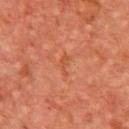image — 15 mm crop, total-body photography; anatomic site — the chest; subject — male, aged around 65.